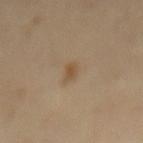{
  "biopsy_status": "not biopsied; imaged during a skin examination",
  "patient": {
    "sex": "female",
    "age_approx": 55
  },
  "lesion_size": {
    "long_diameter_mm_approx": 2.0
  },
  "site": "mid back",
  "image": {
    "source": "total-body photography crop",
    "field_of_view_mm": 15
  },
  "lighting": "cross-polarized"
}